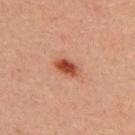biopsy status: catalogued during a skin exam; not biopsied | size: ≈3 mm | location: the back | imaging modality: ~15 mm tile from a whole-body skin photo | tile lighting: cross-polarized | subject: male, in their 30s.